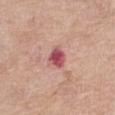The lesion was tiled from a total-body skin photograph and was not biopsied. Cropped from a whole-body photographic skin survey; the tile spans about 15 mm. The lesion's longest dimension is about 2.5 mm. The lesion is on the left thigh. Captured under white-light illumination. A female subject, approximately 65 years of age.